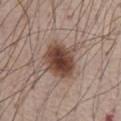Notes:
– biopsy status — total-body-photography surveillance lesion; no biopsy
– subject — male, roughly 65 years of age
– body site — the chest
– diameter — ≈5 mm
– TBP lesion metrics — a lesion area of about 17 mm², an outline eccentricity of about 0.5 (0 = round, 1 = elongated), and two-axis asymmetry of about 0.2; an automated nevus-likeness rating near 100 out of 100 and a detector confidence of about 100 out of 100 that the crop contains a lesion
– image source — 15 mm crop, total-body photography